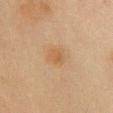notes — no biopsy performed (imaged during a skin exam)
image — 15 mm crop, total-body photography
anatomic site — the chest
subject — female, aged 48–52